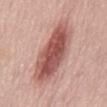No biopsy was performed on this lesion — it was imaged during a full skin examination and was not determined to be concerning.
A 15 mm close-up extracted from a 3D total-body photography capture.
Imaged with white-light lighting.
The subject is a female approximately 65 years of age.
Approximately 9 mm at its widest.
Located on the mid back.